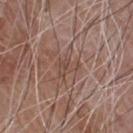| key | value |
|---|---|
| follow-up | catalogued during a skin exam; not biopsied |
| lesion diameter | ≈4 mm |
| body site | the chest |
| subject | male, approximately 80 years of age |
| acquisition | 15 mm crop, total-body photography |
| lighting | white-light |